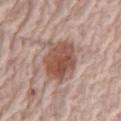Captured during whole-body skin photography for melanoma surveillance; the lesion was not biopsied.
Measured at roughly 5.5 mm in maximum diameter.
A female subject roughly 65 years of age.
The lesion is on the abdomen.
A roughly 15 mm field-of-view crop from a total-body skin photograph.